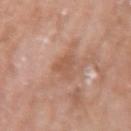<tbp_lesion>
<biopsy_status>not biopsied; imaged during a skin examination</biopsy_status>
<automated_metrics>
  <border_irregularity_0_10>4.0</border_irregularity_0_10>
  <color_variation_0_10>1.0</color_variation_0_10>
  <peripheral_color_asymmetry>0.5</peripheral_color_asymmetry>
</automated_metrics>
<image>
  <source>total-body photography crop</source>
  <field_of_view_mm>15</field_of_view_mm>
</image>
<site>right forearm</site>
<lesion_size>
  <long_diameter_mm_approx>3.0</long_diameter_mm_approx>
</lesion_size>
<patient>
  <sex>female</sex>
  <age_approx>75</age_approx>
</patient>
</tbp_lesion>